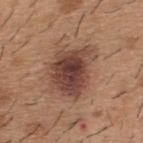Recorded during total-body skin imaging; not selected for excision or biopsy. An algorithmic analysis of the crop reported a lesion area of about 21 mm² and an eccentricity of roughly 0.65. The recorded lesion diameter is about 6 mm. On the upper back. A close-up tile cropped from a whole-body skin photograph, about 15 mm across. A male subject roughly 40 years of age. Imaged with white-light lighting.